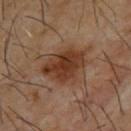No biopsy was performed on this lesion — it was imaged during a full skin examination and was not determined to be concerning. Longest diameter approximately 5 mm. An algorithmic analysis of the crop reported a lesion color around L≈34 a*≈19 b*≈28 in CIELAB, roughly 10 lightness units darker than nearby skin, and a normalized lesion–skin contrast near 9.5. It also reported a border-irregularity rating of about 3.5/10, a within-lesion color-variation index near 4/10, and radial color variation of about 1. And it measured a nevus-likeness score of about 70/100 and a lesion-detection confidence of about 100/100. Imaged with cross-polarized lighting. The lesion is on the upper back. The subject is a male in their mid-60s. A 15 mm close-up extracted from a 3D total-body photography capture.